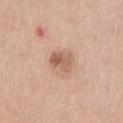{
  "biopsy_status": "not biopsied; imaged during a skin examination",
  "image": {
    "source": "total-body photography crop",
    "field_of_view_mm": 15
  },
  "site": "chest",
  "patient": {
    "sex": "male",
    "age_approx": 60
  }
}